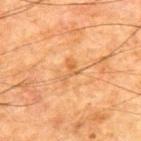Captured during whole-body skin photography for melanoma surveillance; the lesion was not biopsied.
The subject is a male approximately 65 years of age.
This image is a 15 mm lesion crop taken from a total-body photograph.
The lesion is located on the back.